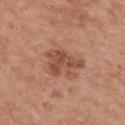The lesion was tiled from a total-body skin photograph and was not biopsied.
The lesion's longest dimension is about 4.5 mm.
A female subject, in their mid- to late 60s.
From the chest.
A region of skin cropped from a whole-body photographic capture, roughly 15 mm wide.